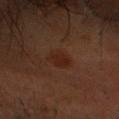follow-up: catalogued during a skin exam; not biopsied
diameter: about 3 mm
anatomic site: the head or neck
image-analysis metrics: an area of roughly 5.5 mm², a shape eccentricity near 0.8, and a shape-asymmetry score of about 0.2 (0 = symmetric); a border-irregularity rating of about 2/10 and a within-lesion color-variation index near 1/10; an automated nevus-likeness rating near 0 out of 100 and a detector confidence of about 100 out of 100 that the crop contains a lesion
illumination: cross-polarized
imaging modality: ~15 mm tile from a whole-body skin photo
subject: male, aged around 50